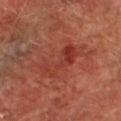Assessment: This lesion was catalogued during total-body skin photography and was not selected for biopsy. Context: A male patient, aged approximately 75. A region of skin cropped from a whole-body photographic capture, roughly 15 mm wide. The lesion is located on the leg. Longest diameter approximately 6 mm. The tile uses cross-polarized illumination.